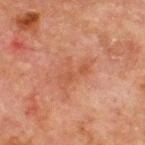| field | value |
|---|---|
| image | 15 mm crop, total-body photography |
| patient | male, approximately 65 years of age |
| anatomic site | the chest |